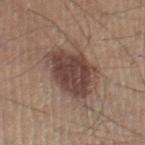<lesion>
  <biopsy_status>not biopsied; imaged during a skin examination</biopsy_status>
  <lighting>white-light</lighting>
  <site>right lower leg</site>
  <patient>
    <sex>male</sex>
    <age_approx>45</age_approx>
  </patient>
  <image>
    <source>total-body photography crop</source>
    <field_of_view_mm>15</field_of_view_mm>
  </image>
  <automated_metrics>
    <area_mm2_approx>21.0</area_mm2_approx>
    <eccentricity>0.75</eccentricity>
    <cielab_L>43</cielab_L>
    <cielab_a>17</cielab_a>
    <cielab_b>23</cielab_b>
    <vs_skin_darker_L>12.0</vs_skin_darker_L>
    <vs_skin_contrast_norm>10.0</vs_skin_contrast_norm>
  </automated_metrics>
  <lesion_size>
    <long_diameter_mm_approx>6.5</long_diameter_mm_approx>
  </lesion_size>
</lesion>